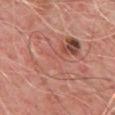Imaged during a routine full-body skin examination; the lesion was not biopsied and no histopathology is available.
A 15 mm close-up extracted from a 3D total-body photography capture.
Located on the front of the torso.
Measured at roughly 8.5 mm in maximum diameter.
Captured under cross-polarized illumination.
A male patient aged around 50.
An algorithmic analysis of the crop reported a footprint of about 40 mm², an outline eccentricity of about 0.7 (0 = round, 1 = elongated), and two-axis asymmetry of about 0.25. And it measured about 5 CIELAB-L* units darker than the surrounding skin and a normalized border contrast of about 3.5. The software also gave a border-irregularity index near 4/10, a color-variation rating of about 9.5/10, and radial color variation of about 3.5.